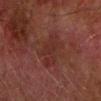Assessment:
The lesion was photographed on a routine skin check and not biopsied; there is no pathology result.
Background:
Located on the right forearm. The subject is a male aged around 75. A region of skin cropped from a whole-body photographic capture, roughly 15 mm wide.